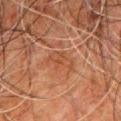No biopsy was performed on this lesion — it was imaged during a full skin examination and was not determined to be concerning. Captured under cross-polarized illumination. From the chest. The subject is a male in their 80s. This image is a 15 mm lesion crop taken from a total-body photograph. Automated tile analysis of the lesion measured border irregularity of about 4.5 on a 0–10 scale, a within-lesion color-variation index near 2.5/10, and a peripheral color-asymmetry measure near 0.5. The lesion's longest dimension is about 4 mm.